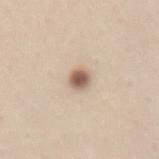{"biopsy_status": "not biopsied; imaged during a skin examination", "site": "lower back", "image": {"source": "total-body photography crop", "field_of_view_mm": 15}, "patient": {"sex": "female", "age_approx": 20}}